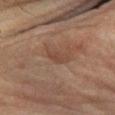Recorded during total-body skin imaging; not selected for excision or biopsy. A 15 mm close-up tile from a total-body photography series done for melanoma screening. A male patient aged 53–57. From the right lower leg. Captured under cross-polarized illumination. About 3.5 mm across.